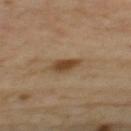On the upper back. Measured at roughly 3.5 mm in maximum diameter. The tile uses cross-polarized illumination. A close-up tile cropped from a whole-body skin photograph, about 15 mm across. The patient is a female in their mid- to late 40s.